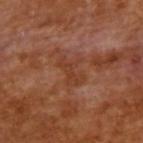This lesion was catalogued during total-body skin photography and was not selected for biopsy. The tile uses cross-polarized illumination. The lesion's longest dimension is about 3.5 mm. A male subject, in their mid-60s. This image is a 15 mm lesion crop taken from a total-body photograph.